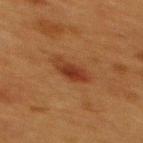Assessment:
Imaged during a routine full-body skin examination; the lesion was not biopsied and no histopathology is available.
Background:
The recorded lesion diameter is about 3.5 mm. A region of skin cropped from a whole-body photographic capture, roughly 15 mm wide. The total-body-photography lesion software estimated a footprint of about 5.5 mm², an outline eccentricity of about 0.85 (0 = round, 1 = elongated), and two-axis asymmetry of about 0.25. The analysis additionally found an average lesion color of about L≈30 a*≈22 b*≈29 (CIELAB), roughly 8 lightness units darker than nearby skin, and a lesion-to-skin contrast of about 8.5 (normalized; higher = more distinct). And it measured a border-irregularity rating of about 2.5/10, a within-lesion color-variation index near 4/10, and radial color variation of about 1.5. It also reported a classifier nevus-likeness of about 100/100 and lesion-presence confidence of about 100/100. The patient is a female aged approximately 40. Imaged with cross-polarized lighting. The lesion is on the upper back.